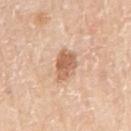Recorded during total-body skin imaging; not selected for excision or biopsy.